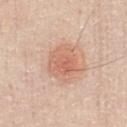Q: Is there a histopathology result?
A: catalogued during a skin exam; not biopsied
Q: What is the anatomic site?
A: the chest
Q: What lighting was used for the tile?
A: white-light illumination
Q: How large is the lesion?
A: about 4.5 mm
Q: What is the imaging modality?
A: total-body-photography crop, ~15 mm field of view
Q: What are the patient's age and sex?
A: male, aged 33–37